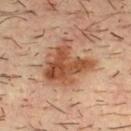  biopsy_status: not biopsied; imaged during a skin examination
  lighting: cross-polarized
  patient:
    sex: male
    age_approx: 35
  image:
    source: total-body photography crop
    field_of_view_mm: 15
  lesion_size:
    long_diameter_mm_approx: 6.0
  site: chest